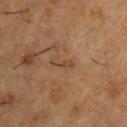The lesion was tiled from a total-body skin photograph and was not biopsied. A 15 mm crop from a total-body photograph taken for skin-cancer surveillance. A male patient, approximately 55 years of age. Approximately 3 mm at its widest. Captured under cross-polarized illumination. The lesion is on the chest.